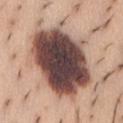{
  "biopsy_status": "not biopsied; imaged during a skin examination",
  "image": {
    "source": "total-body photography crop",
    "field_of_view_mm": 15
  },
  "site": "abdomen",
  "patient": {
    "sex": "female",
    "age_approx": 25
  }
}